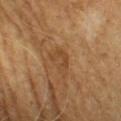notes: catalogued during a skin exam; not biopsied | size: about 3.5 mm | anatomic site: the chest | subject: male, aged 58 to 62 | automated lesion analysis: an average lesion color of about L≈43 a*≈19 b*≈34 (CIELAB); a border-irregularity rating of about 6/10, internal color variation of about 0.5 on a 0–10 scale, and radial color variation of about 0; a classifier nevus-likeness of about 0/100 | illumination: cross-polarized | image: ~15 mm crop, total-body skin-cancer survey.